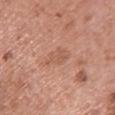This lesion was catalogued during total-body skin photography and was not selected for biopsy. A female subject aged 58 to 62. A region of skin cropped from a whole-body photographic capture, roughly 15 mm wide. The lesion is on the upper back.